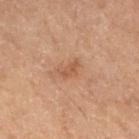The lesion is located on the right thigh. Captured under cross-polarized illumination. This image is a 15 mm lesion crop taken from a total-body photograph. A male subject approximately 70 years of age. Measured at roughly 3 mm in maximum diameter.